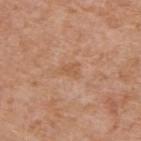<record>
<biopsy_status>not biopsied; imaged during a skin examination</biopsy_status>
<site>upper back</site>
<lesion_size>
  <long_diameter_mm_approx>2.5</long_diameter_mm_approx>
</lesion_size>
<patient>
  <sex>male</sex>
  <age_approx>60</age_approx>
</patient>
<image>
  <source>total-body photography crop</source>
  <field_of_view_mm>15</field_of_view_mm>
</image>
</record>